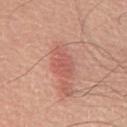Assessment:
Imaged during a routine full-body skin examination; the lesion was not biopsied and no histopathology is available.
Image and clinical context:
The subject is a male aged 38–42. A 15 mm close-up tile from a total-body photography series done for melanoma screening. The lesion is on the chest.